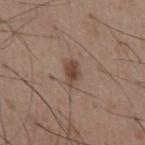The lesion was photographed on a routine skin check and not biopsied; there is no pathology result.
Located on the front of the torso.
The subject is a male aged approximately 55.
A region of skin cropped from a whole-body photographic capture, roughly 15 mm wide.